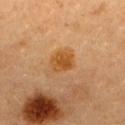The tile uses cross-polarized illumination. The total-body-photography lesion software estimated a lesion-to-skin contrast of about 9 (normalized; higher = more distinct). A region of skin cropped from a whole-body photographic capture, roughly 15 mm wide. The lesion is located on the upper back. A female patient, approximately 60 years of age.